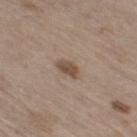Imaged during a routine full-body skin examination; the lesion was not biopsied and no histopathology is available.
The total-body-photography lesion software estimated an area of roughly 3.5 mm², an eccentricity of roughly 0.8, and two-axis asymmetry of about 0.2. It also reported a lesion color around L≈49 a*≈16 b*≈27 in CIELAB, a lesion–skin lightness drop of about 11, and a normalized lesion–skin contrast near 8.5. And it measured a detector confidence of about 100 out of 100 that the crop contains a lesion.
From the right thigh.
A 15 mm crop from a total-body photograph taken for skin-cancer surveillance.
The subject is a male aged 68 to 72.